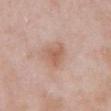biopsy status = imaged on a skin check; not biopsied | imaging modality = total-body-photography crop, ~15 mm field of view | tile lighting = white-light | subject = male, in their mid- to late 50s | automated metrics = an average lesion color of about L≈59 a*≈21 b*≈29 (CIELAB), roughly 9 lightness units darker than nearby skin, and a normalized border contrast of about 6.5; border irregularity of about 4 on a 0–10 scale and internal color variation of about 2.5 on a 0–10 scale; a nevus-likeness score of about 35/100 and a detector confidence of about 100 out of 100 that the crop contains a lesion | lesion diameter = ~3.5 mm (longest diameter) | body site = the abdomen.